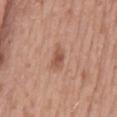The lesion was photographed on a routine skin check and not biopsied; there is no pathology result.
Captured under white-light illumination.
A male subject, aged 53–57.
Cropped from a whole-body photographic skin survey; the tile spans about 15 mm.
Longest diameter approximately 3 mm.
An algorithmic analysis of the crop reported a lesion area of about 4.5 mm². The software also gave a detector confidence of about 100 out of 100 that the crop contains a lesion.
From the back.